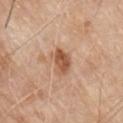Assessment: Imaged during a routine full-body skin examination; the lesion was not biopsied and no histopathology is available. Background: The lesion is on the front of the torso. A 15 mm close-up tile from a total-body photography series done for melanoma screening. A male subject aged around 80. The total-body-photography lesion software estimated a nevus-likeness score of about 70/100 and lesion-presence confidence of about 100/100. Imaged with white-light lighting.